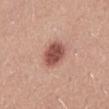Clinical impression:
Captured during whole-body skin photography for melanoma surveillance; the lesion was not biopsied.
Image and clinical context:
Located on the mid back. The subject is a male aged approximately 25. Cropped from a total-body skin-imaging series; the visible field is about 15 mm. Automated tile analysis of the lesion measured a footprint of about 9 mm², a shape eccentricity near 0.45, and a symmetry-axis asymmetry near 0.1. It also reported a lesion-to-skin contrast of about 10 (normalized; higher = more distinct). Captured under white-light illumination.